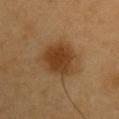workup=imaged on a skin check; not biopsied
tile lighting=cross-polarized illumination
automated lesion analysis=border irregularity of about 2.5 on a 0–10 scale, internal color variation of about 3.5 on a 0–10 scale, and a peripheral color-asymmetry measure near 1; a nevus-likeness score of about 100/100
image=~15 mm crop, total-body skin-cancer survey
subject=male, in their 60s
body site=the chest